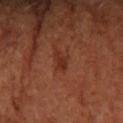Assessment:
Captured during whole-body skin photography for melanoma surveillance; the lesion was not biopsied.
Clinical summary:
Approximately 3 mm at its widest. Imaged with cross-polarized lighting. Located on the right forearm. A female subject aged 63 to 67. Cropped from a total-body skin-imaging series; the visible field is about 15 mm.